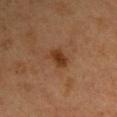Impression: Imaged during a routine full-body skin examination; the lesion was not biopsied and no histopathology is available. Context: A female subject in their 40s. A region of skin cropped from a whole-body photographic capture, roughly 15 mm wide. Captured under cross-polarized illumination. Located on the left upper arm. Longest diameter approximately 3 mm.